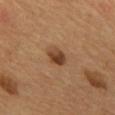The lesion was photographed on a routine skin check and not biopsied; there is no pathology result. From the mid back. The lesion's longest dimension is about 2.5 mm. A 15 mm crop from a total-body photograph taken for skin-cancer surveillance. An algorithmic analysis of the crop reported a footprint of about 4.5 mm², an outline eccentricity of about 0.5 (0 = round, 1 = elongated), and two-axis asymmetry of about 0.2. The analysis additionally found a nevus-likeness score of about 90/100. A male subject, about 55 years old. This is a cross-polarized tile.